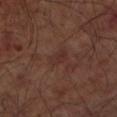Impression: The lesion was tiled from a total-body skin photograph and was not biopsied. Background: Imaged with cross-polarized lighting. This image is a 15 mm lesion crop taken from a total-body photograph. The lesion's longest dimension is about 2.5 mm. The patient is a male aged 63 to 67. Located on the left forearm.